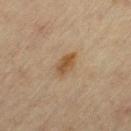tile lighting: cross-polarized
patient: female, aged approximately 65
location: the left thigh
image-analysis metrics: a shape-asymmetry score of about 0.2 (0 = symmetric); an automated nevus-likeness rating near 85 out of 100 and a lesion-detection confidence of about 100/100
image source: 15 mm crop, total-body photography